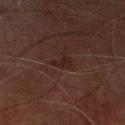Notes:
- follow-up: total-body-photography surveillance lesion; no biopsy
- patient: male, in their 70s
- TBP lesion metrics: roughly 4 lightness units darker than nearby skin and a normalized lesion–skin contrast near 6; an automated nevus-likeness rating near 0 out of 100 and a detector confidence of about 100 out of 100 that the crop contains a lesion
- acquisition: total-body-photography crop, ~15 mm field of view
- lesion diameter: ~2.5 mm (longest diameter)
- body site: the right lower leg